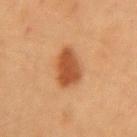Impression: Part of a total-body skin-imaging series; this lesion was reviewed on a skin check and was not flagged for biopsy.